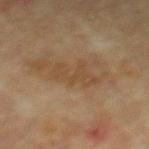Clinical summary: A male patient aged 63–67. Cropped from a total-body skin-imaging series; the visible field is about 15 mm. Automated image analysis of the tile measured a lesion area of about 7 mm² and an eccentricity of roughly 0.9. Approximately 5 mm at its widest. From the mid back. Imaged with cross-polarized lighting.